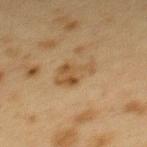Notes:
– biopsy status: catalogued during a skin exam; not biopsied
– image-analysis metrics: an automated nevus-likeness rating near 15 out of 100 and a lesion-detection confidence of about 100/100
– subject: female, aged 38–42
– tile lighting: cross-polarized
– body site: the upper back
– image source: 15 mm crop, total-body photography
– diameter: ~4 mm (longest diameter)